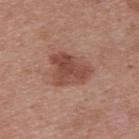notes — catalogued during a skin exam; not biopsied
site — the back
illumination — white-light illumination
patient — female, roughly 45 years of age
TBP lesion metrics — a detector confidence of about 100 out of 100 that the crop contains a lesion
acquisition — total-body-photography crop, ~15 mm field of view
lesion size — ~5 mm (longest diameter)